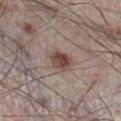{"biopsy_status": "not biopsied; imaged during a skin examination", "image": {"source": "total-body photography crop", "field_of_view_mm": 15}, "automated_metrics": {"cielab_L": 45, "cielab_a": 17, "cielab_b": 23, "vs_skin_darker_L": 13.0, "vs_skin_contrast_norm": 10.0, "nevus_likeness_0_100": 95}, "lesion_size": {"long_diameter_mm_approx": 3.0}, "patient": {"sex": "male", "age_approx": 60}, "site": "right lower leg"}